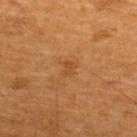Captured during whole-body skin photography for melanoma surveillance; the lesion was not biopsied.
Measured at roughly 2 mm in maximum diameter.
A male patient, in their 60s.
This image is a 15 mm lesion crop taken from a total-body photograph.
The lesion is located on the upper back.
Automated tile analysis of the lesion measured a mean CIELAB color near L≈44 a*≈23 b*≈38, roughly 6 lightness units darker than nearby skin, and a lesion-to-skin contrast of about 5 (normalized; higher = more distinct).